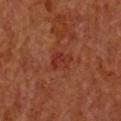The lesion was photographed on a routine skin check and not biopsied; there is no pathology result.
A female patient aged 63–67.
About 3 mm across.
The tile uses cross-polarized illumination.
Automated tile analysis of the lesion measured a lesion area of about 5 mm², an outline eccentricity of about 0.75 (0 = round, 1 = elongated), and a symmetry-axis asymmetry near 0.35. And it measured an average lesion color of about L≈34 a*≈30 b*≈30 (CIELAB), about 6 CIELAB-L* units darker than the surrounding skin, and a normalized lesion–skin contrast near 6. And it measured border irregularity of about 3 on a 0–10 scale, internal color variation of about 4 on a 0–10 scale, and radial color variation of about 1.5.
The lesion is located on the chest.
A 15 mm crop from a total-body photograph taken for skin-cancer surveillance.